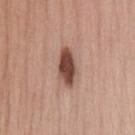<lesion>
<biopsy_status>not biopsied; imaged during a skin examination</biopsy_status>
<patient>
  <sex>female</sex>
  <age_approx>40</age_approx>
</patient>
<lesion_size>
  <long_diameter_mm_approx>4.5</long_diameter_mm_approx>
</lesion_size>
<lighting>white-light</lighting>
<image>
  <source>total-body photography crop</source>
  <field_of_view_mm>15</field_of_view_mm>
</image>
<automated_metrics>
  <nevus_likeness_0_100>95</nevus_likeness_0_100>
  <lesion_detection_confidence_0_100>100</lesion_detection_confidence_0_100>
</automated_metrics>
<site>lower back</site>
</lesion>